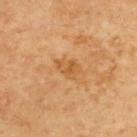Q: Is there a histopathology result?
A: imaged on a skin check; not biopsied
Q: What kind of image is this?
A: ~15 mm crop, total-body skin-cancer survey
Q: What is the anatomic site?
A: the upper back
Q: Automated lesion metrics?
A: an area of roughly 5 mm², a shape eccentricity near 0.75, and two-axis asymmetry of about 0.35; a classifier nevus-likeness of about 0/100 and a lesion-detection confidence of about 100/100
Q: What is the lesion's diameter?
A: ~3 mm (longest diameter)
Q: Patient demographics?
A: male, approximately 70 years of age
Q: Illumination type?
A: cross-polarized illumination